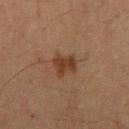{"biopsy_status": "not biopsied; imaged during a skin examination"}